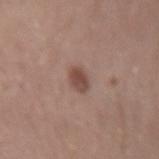notes: imaged on a skin check; not biopsied | tile lighting: white-light illumination | location: the arm | subject: male, aged around 30 | lesion size: ~2.5 mm (longest diameter) | automated metrics: a footprint of about 4 mm², a shape eccentricity near 0.8, and two-axis asymmetry of about 0.15; an automated nevus-likeness rating near 90 out of 100 and a detector confidence of about 100 out of 100 that the crop contains a lesion | image: ~15 mm crop, total-body skin-cancer survey.